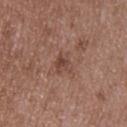On the back.
Imaged with white-light lighting.
A roughly 15 mm field-of-view crop from a total-body skin photograph.
The recorded lesion diameter is about 2.5 mm.
A male subject, about 50 years old.
The total-body-photography lesion software estimated a border-irregularity index near 3.5/10. It also reported an automated nevus-likeness rating near 0 out of 100 and a detector confidence of about 100 out of 100 that the crop contains a lesion.